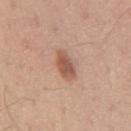Assessment:
The lesion was tiled from a total-body skin photograph and was not biopsied.
Clinical summary:
The recorded lesion diameter is about 3.5 mm. The lesion is on the mid back. A male patient aged 53 to 57. The total-body-photography lesion software estimated an eccentricity of roughly 0.75 and a symmetry-axis asymmetry near 0.15. It also reported an average lesion color of about L≈55 a*≈22 b*≈29 (CIELAB), about 12 CIELAB-L* units darker than the surrounding skin, and a normalized border contrast of about 8. A 15 mm close-up extracted from a 3D total-body photography capture.